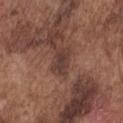{"biopsy_status": "not biopsied; imaged during a skin examination", "patient": {"sex": "male", "age_approx": 75}, "image": {"source": "total-body photography crop", "field_of_view_mm": 15}, "site": "front of the torso"}